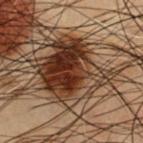Notes:
- workup · total-body-photography surveillance lesion; no biopsy
- lesion diameter · about 7 mm
- patient · male, aged approximately 55
- site · the chest
- automated metrics · a mean CIELAB color near L≈28 a*≈16 b*≈24 and roughly 14 lightness units darker than nearby skin; border irregularity of about 3 on a 0–10 scale and a within-lesion color-variation index near 10/10; a classifier nevus-likeness of about 85/100 and a lesion-detection confidence of about 100/100
- acquisition · ~15 mm crop, total-body skin-cancer survey
- tile lighting · cross-polarized illumination